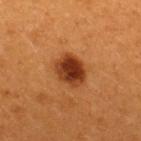<record>
  <biopsy_status>not biopsied; imaged during a skin examination</biopsy_status>
  <patient>
    <sex>female</sex>
    <age_approx>35</age_approx>
  </patient>
  <site>upper back</site>
  <automated_metrics>
    <cielab_L>38</cielab_L>
    <cielab_a>28</cielab_a>
    <cielab_b>38</cielab_b>
    <vs_skin_darker_L>16.0</vs_skin_darker_L>
  </automated_metrics>
  <image>
    <source>total-body photography crop</source>
    <field_of_view_mm>15</field_of_view_mm>
  </image>
  <lesion_size>
    <long_diameter_mm_approx>4.0</long_diameter_mm_approx>
  </lesion_size>
</record>